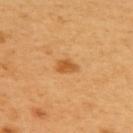On the upper back. Automated tile analysis of the lesion measured a shape eccentricity near 0.7 and a shape-asymmetry score of about 0.35 (0 = symmetric). And it measured a mean CIELAB color near L≈57 a*≈25 b*≈47, a lesion–skin lightness drop of about 11, and a normalized border contrast of about 7.5. The tile uses cross-polarized illumination. A roughly 15 mm field-of-view crop from a total-body skin photograph. A female subject aged 53–57. Measured at roughly 2.5 mm in maximum diameter.